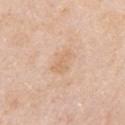| feature | finding |
|---|---|
| follow-up | no biopsy performed (imaged during a skin exam) |
| imaging modality | ~15 mm tile from a whole-body skin photo |
| location | the right upper arm |
| lesion diameter | about 3.5 mm |
| lighting | white-light illumination |
| patient | female, aged approximately 60 |
| image-analysis metrics | an average lesion color of about L≈69 a*≈18 b*≈34 (CIELAB), roughly 6 lightness units darker than nearby skin, and a normalized border contrast of about 4.5; border irregularity of about 2.5 on a 0–10 scale, a within-lesion color-variation index near 2/10, and radial color variation of about 0.5; a nevus-likeness score of about 0/100 |